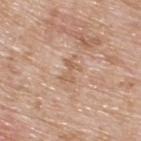The lesion was photographed on a routine skin check and not biopsied; there is no pathology result. About 3 mm across. The tile uses white-light illumination. The patient is a male in their 60s. The lesion is located on the upper back. The lesion-visualizer software estimated a footprint of about 4 mm², a shape eccentricity near 0.8, and two-axis asymmetry of about 0.5. The analysis additionally found a mean CIELAB color near L≈60 a*≈18 b*≈31, a lesion–skin lightness drop of about 7, and a normalized border contrast of about 5.5. The software also gave a nevus-likeness score of about 0/100. A region of skin cropped from a whole-body photographic capture, roughly 15 mm wide.